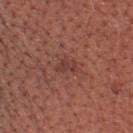This lesion was catalogued during total-body skin photography and was not selected for biopsy. A male patient, aged 43 to 47. The lesion is on the head or neck. Captured under white-light illumination. Measured at roughly 2.5 mm in maximum diameter. Cropped from a whole-body photographic skin survey; the tile spans about 15 mm. Automated image analysis of the tile measured a border-irregularity rating of about 4.5/10, internal color variation of about 1 on a 0–10 scale, and radial color variation of about 0. The analysis additionally found a detector confidence of about 100 out of 100 that the crop contains a lesion.